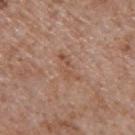{"biopsy_status": "not biopsied; imaged during a skin examination", "lighting": "white-light", "lesion_size": {"long_diameter_mm_approx": 3.5}, "patient": {"sex": "male", "age_approx": 65}, "image": {"source": "total-body photography crop", "field_of_view_mm": 15}, "automated_metrics": {"area_mm2_approx": 4.0, "eccentricity": 0.9, "shape_asymmetry": 0.45, "border_irregularity_0_10": 6.0, "color_variation_0_10": 1.5, "peripheral_color_asymmetry": 0.5, "nevus_likeness_0_100": 0, "lesion_detection_confidence_0_100": 100}, "site": "mid back"}